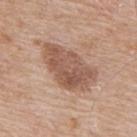workup: no biopsy performed (imaged during a skin exam)
imaging modality: ~15 mm tile from a whole-body skin photo
patient: male, in their mid- to late 60s
tile lighting: white-light
site: the upper back